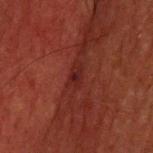Q: Is there a histopathology result?
A: total-body-photography surveillance lesion; no biopsy
Q: What is the imaging modality?
A: total-body-photography crop, ~15 mm field of view
Q: What are the patient's age and sex?
A: male, about 60 years old
Q: What is the anatomic site?
A: the head or neck
Q: Lesion size?
A: ≈3 mm
Q: How was the tile lit?
A: cross-polarized illumination